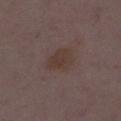Part of a total-body skin-imaging series; this lesion was reviewed on a skin check and was not flagged for biopsy. About 3.5 mm across. An algorithmic analysis of the crop reported a border-irregularity rating of about 2.5/10. Captured under white-light illumination. A region of skin cropped from a whole-body photographic capture, roughly 15 mm wide. The patient is a female in their mid-30s. On the right thigh.